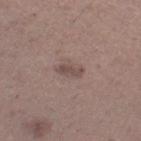The lesion was tiled from a total-body skin photograph and was not biopsied.
A region of skin cropped from a whole-body photographic capture, roughly 15 mm wide.
The subject is a female aged around 30.
From the right thigh.